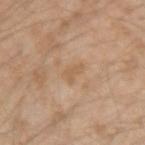Impression:
Recorded during total-body skin imaging; not selected for excision or biopsy.
Clinical summary:
Cropped from a total-body skin-imaging series; the visible field is about 15 mm. The lesion's longest dimension is about 2.5 mm. Captured under white-light illumination. An algorithmic analysis of the crop reported a lesion area of about 3.5 mm² and a shape-asymmetry score of about 0.4 (0 = symmetric). The software also gave a border-irregularity rating of about 4/10, a within-lesion color-variation index near 0.5/10, and a peripheral color-asymmetry measure near 0. And it measured a nevus-likeness score of about 0/100 and a lesion-detection confidence of about 100/100. A male patient in their mid- to late 30s. Located on the arm.